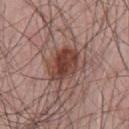Part of a total-body skin-imaging series; this lesion was reviewed on a skin check and was not flagged for biopsy. A lesion tile, about 15 mm wide, cut from a 3D total-body photograph. Automated tile analysis of the lesion measured a footprint of about 12 mm². And it measured a lesion color around L≈42 a*≈22 b*≈24 in CIELAB, roughly 12 lightness units darker than nearby skin, and a lesion-to-skin contrast of about 9.5 (normalized; higher = more distinct). The lesion is on the chest. The patient is a male aged 63–67. Captured under white-light illumination. Longest diameter approximately 4.5 mm.